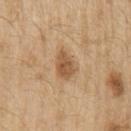Notes:
- lighting · white-light illumination
- subject · male, about 70 years old
- lesion diameter · about 3.5 mm
- image · ~15 mm crop, total-body skin-cancer survey
- body site · the left upper arm